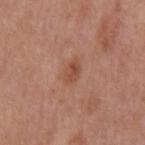workup = imaged on a skin check; not biopsied
lighting = white-light illumination
automated metrics = an eccentricity of roughly 0.85 and two-axis asymmetry of about 0.3; a border-irregularity rating of about 2.5/10, a within-lesion color-variation index near 4/10, and a peripheral color-asymmetry measure near 1.5
subject = male, about 70 years old
site = the back
imaging modality = total-body-photography crop, ~15 mm field of view